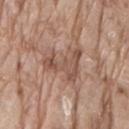Impression:
This lesion was catalogued during total-body skin photography and was not selected for biopsy.
Image and clinical context:
A close-up tile cropped from a whole-body skin photograph, about 15 mm across. A male subject, aged 78 to 82. On the lower back. Longest diameter approximately 5 mm. Captured under white-light illumination.